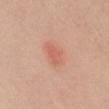Clinical impression:
Recorded during total-body skin imaging; not selected for excision or biopsy.
Clinical summary:
This is a white-light tile. A lesion tile, about 15 mm wide, cut from a 3D total-body photograph. The lesion-visualizer software estimated a footprint of about 5 mm², a shape eccentricity near 0.85, and a symmetry-axis asymmetry near 0.25. The software also gave a mean CIELAB color near L≈61 a*≈26 b*≈31 and a normalized border contrast of about 5. And it measured border irregularity of about 2.5 on a 0–10 scale, a within-lesion color-variation index near 2.5/10, and a peripheral color-asymmetry measure near 1. It also reported a lesion-detection confidence of about 100/100. The subject is a female about 40 years old. The lesion is on the mid back.